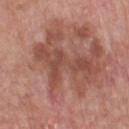Impression: Part of a total-body skin-imaging series; this lesion was reviewed on a skin check and was not flagged for biopsy. Acquisition and patient details: On the front of the torso. Cropped from a whole-body photographic skin survey; the tile spans about 15 mm. Captured under white-light illumination. Longest diameter approximately 8 mm. A male patient, aged 63 to 67.